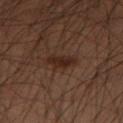Recorded during total-body skin imaging; not selected for excision or biopsy. A close-up tile cropped from a whole-body skin photograph, about 15 mm across. The lesion is located on the leg. A male subject, aged around 55.